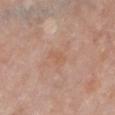Automated tile analysis of the lesion measured a footprint of about 4 mm² and a shape eccentricity near 0.7. It also reported a nevus-likeness score of about 0/100.
The lesion's longest dimension is about 2.5 mm.
The patient is a female in their mid- to late 60s.
On the chest.
A region of skin cropped from a whole-body photographic capture, roughly 15 mm wide.
This is a white-light tile.